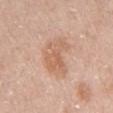| key | value |
|---|---|
| notes | catalogued during a skin exam; not biopsied |
| site | the left upper arm |
| TBP lesion metrics | a border-irregularity rating of about 4.5/10, internal color variation of about 3.5 on a 0–10 scale, and a peripheral color-asymmetry measure near 1; a classifier nevus-likeness of about 5/100 and a lesion-detection confidence of about 100/100 |
| subject | female, aged 48–52 |
| tile lighting | white-light |
| lesion diameter | about 5 mm |
| imaging modality | ~15 mm tile from a whole-body skin photo |